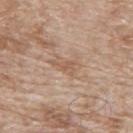The patient is a male in their 80s. Cropped from a whole-body photographic skin survey; the tile spans about 15 mm. From the back.